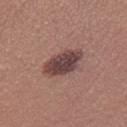<tbp_lesion>
  <biopsy_status>not biopsied; imaged during a skin examination</biopsy_status>
  <site>right thigh</site>
  <patient>
    <sex>female</sex>
    <age_approx>25</age_approx>
  </patient>
  <image>
    <source>total-body photography crop</source>
    <field_of_view_mm>15</field_of_view_mm>
  </image>
  <lighting>white-light</lighting>
</tbp_lesion>